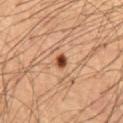follow-up: imaged on a skin check; not biopsied
acquisition: total-body-photography crop, ~15 mm field of view
subject: male, roughly 65 years of age
location: the mid back